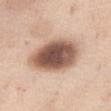Impression:
No biopsy was performed on this lesion — it was imaged during a full skin examination and was not determined to be concerning.
Image and clinical context:
From the abdomen. This is a white-light tile. The subject is a female roughly 55 years of age. The recorded lesion diameter is about 7 mm. A 15 mm close-up tile from a total-body photography series done for melanoma screening. The total-body-photography lesion software estimated an area of roughly 25 mm², a shape eccentricity near 0.75, and a symmetry-axis asymmetry near 0.15. It also reported an average lesion color of about L≈56 a*≈18 b*≈27 (CIELAB), roughly 20 lightness units darker than nearby skin, and a normalized lesion–skin contrast near 12.5. The analysis additionally found a border-irregularity rating of about 1.5/10, a color-variation rating of about 7/10, and a peripheral color-asymmetry measure near 1.5. It also reported a nevus-likeness score of about 90/100 and lesion-presence confidence of about 100/100.